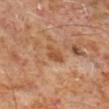workup: total-body-photography surveillance lesion; no biopsy
image: ~15 mm crop, total-body skin-cancer survey
illumination: cross-polarized illumination
site: the right lower leg
lesion size: about 2.5 mm
patient: male, approximately 60 years of age
automated lesion analysis: a lesion area of about 4 mm², an outline eccentricity of about 0.6 (0 = round, 1 = elongated), and two-axis asymmetry of about 0.4; a within-lesion color-variation index near 2/10 and peripheral color asymmetry of about 0.5; a classifier nevus-likeness of about 0/100